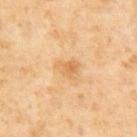Imaged during a routine full-body skin examination; the lesion was not biopsied and no histopathology is available. The subject is a male approximately 65 years of age. Cropped from a whole-body photographic skin survey; the tile spans about 15 mm. About 3 mm across. Captured under cross-polarized illumination.